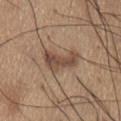No biopsy was performed on this lesion — it was imaged during a full skin examination and was not determined to be concerning. A male patient, in their mid-60s. A 15 mm crop from a total-body photograph taken for skin-cancer surveillance. About 4.5 mm across. From the abdomen.